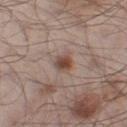This lesion was catalogued during total-body skin photography and was not selected for biopsy.
About 3 mm across.
Imaged with white-light lighting.
A male patient, aged 53–57.
From the left thigh.
Automated tile analysis of the lesion measured an area of roughly 4.5 mm², an eccentricity of roughly 0.65, and two-axis asymmetry of about 0.2.
Cropped from a total-body skin-imaging series; the visible field is about 15 mm.